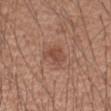notes: catalogued during a skin exam; not biopsied
lesion diameter: about 3 mm
imaging modality: 15 mm crop, total-body photography
patient: male, approximately 55 years of age
tile lighting: white-light
automated metrics: a nevus-likeness score of about 65/100